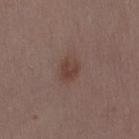Assessment:
The lesion was tiled from a total-body skin photograph and was not biopsied.
Acquisition and patient details:
A female subject in their 50s. The lesion is on the right thigh. A roughly 15 mm field-of-view crop from a total-body skin photograph. The recorded lesion diameter is about 3 mm.